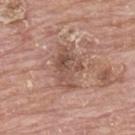The lesion was photographed on a routine skin check and not biopsied; there is no pathology result.
A 15 mm close-up tile from a total-body photography series done for melanoma screening.
The patient is a female aged 63–67.
On the chest.
Captured under white-light illumination.